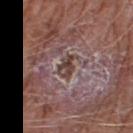The lesion was tiled from a total-body skin photograph and was not biopsied.
Captured under white-light illumination.
The lesion is located on the right thigh.
The lesion-visualizer software estimated an area of roughly 4.5 mm². The analysis additionally found an automated nevus-likeness rating near 0 out of 100.
A lesion tile, about 15 mm wide, cut from a 3D total-body photograph.
Longest diameter approximately 3 mm.
The subject is a male aged around 65.